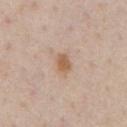lesion size: ≈2.5 mm | lighting: white-light illumination | imaging modality: 15 mm crop, total-body photography | site: the chest | patient: male, about 60 years old.